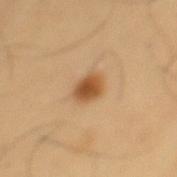Assessment: No biopsy was performed on this lesion — it was imaged during a full skin examination and was not determined to be concerning. Clinical summary: The tile uses cross-polarized illumination. Cropped from a whole-body photographic skin survey; the tile spans about 15 mm. The subject is a male in their mid- to late 50s. Measured at roughly 3 mm in maximum diameter. The lesion is located on the mid back. Automated tile analysis of the lesion measured an area of roughly 6 mm² and an outline eccentricity of about 0.6 (0 = round, 1 = elongated). And it measured a border-irregularity index near 2/10, internal color variation of about 4 on a 0–10 scale, and a peripheral color-asymmetry measure near 1.